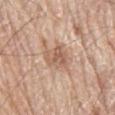workup: catalogued during a skin exam; not biopsied | image source: ~15 mm tile from a whole-body skin photo | subject: male, aged around 80 | body site: the mid back.